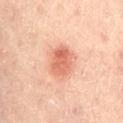Image and clinical context:
The lesion is on the lower back. A male patient, approximately 65 years of age. About 4 mm across. Cropped from a whole-body photographic skin survey; the tile spans about 15 mm. This is a cross-polarized tile.